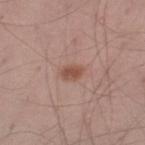– follow-up — catalogued during a skin exam; not biopsied
– patient — male, aged 28–32
– anatomic site — the leg
– image source — ~15 mm crop, total-body skin-cancer survey